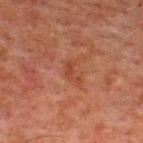| field | value |
|---|---|
| follow-up | total-body-photography surveillance lesion; no biopsy |
| anatomic site | the upper back |
| diameter | ≈3 mm |
| lighting | cross-polarized illumination |
| subject | male, in their 60s |
| imaging modality | total-body-photography crop, ~15 mm field of view |